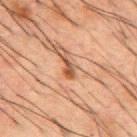Part of a total-body skin-imaging series; this lesion was reviewed on a skin check and was not flagged for biopsy.
A male subject about 50 years old.
This is a cross-polarized tile.
A region of skin cropped from a whole-body photographic capture, roughly 15 mm wide.
An algorithmic analysis of the crop reported an area of roughly 2.5 mm² and two-axis asymmetry of about 0.45. The analysis additionally found a mean CIELAB color near L≈41 a*≈20 b*≈28, roughly 11 lightness units darker than nearby skin, and a normalized lesion–skin contrast near 9. It also reported a border-irregularity index near 4.5/10, a within-lesion color-variation index near 0/10, and a peripheral color-asymmetry measure near 0.
The lesion is located on the mid back.